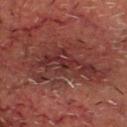Case summary:
• follow-up: no biopsy performed (imaged during a skin exam)
• subject: male, aged 58–62
• lighting: cross-polarized
• size: ~11 mm (longest diameter)
• acquisition: ~15 mm tile from a whole-body skin photo
• image-analysis metrics: an area of roughly 37 mm², an outline eccentricity of about 0.85 (0 = round, 1 = elongated), and a shape-asymmetry score of about 0.4 (0 = symmetric); a nevus-likeness score of about 0/100
• location: the head or neck